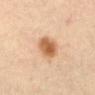The lesion was tiled from a total-body skin photograph and was not biopsied.
Cropped from a total-body skin-imaging series; the visible field is about 15 mm.
The lesion's longest dimension is about 4 mm.
The subject is a male aged 68–72.
From the back.